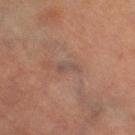A close-up tile cropped from a whole-body skin photograph, about 15 mm across.
The lesion is on the leg.
A female subject, approximately 70 years of age.
Measured at roughly 2.5 mm in maximum diameter.